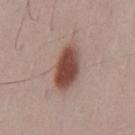Clinical summary:
The total-body-photography lesion software estimated a mean CIELAB color near L≈47 a*≈21 b*≈24, about 16 CIELAB-L* units darker than the surrounding skin, and a lesion-to-skin contrast of about 11.5 (normalized; higher = more distinct). The software also gave lesion-presence confidence of about 100/100. From the mid back. The recorded lesion diameter is about 5.5 mm. The tile uses white-light illumination. A region of skin cropped from a whole-body photographic capture, roughly 15 mm wide. A male subject aged 48 to 52.